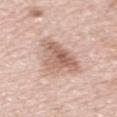Recorded during total-body skin imaging; not selected for excision or biopsy. Automated image analysis of the tile measured a border-irregularity rating of about 4.5/10, internal color variation of about 5.5 on a 0–10 scale, and a peripheral color-asymmetry measure near 2. Imaged with white-light lighting. Cropped from a whole-body photographic skin survey; the tile spans about 15 mm. The lesion is located on the left upper arm. A female subject, roughly 50 years of age. Longest diameter approximately 5 mm.